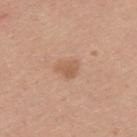<record>
  <biopsy_status>not biopsied; imaged during a skin examination</biopsy_status>
  <lighting>white-light</lighting>
  <patient>
    <sex>male</sex>
    <age_approx>25</age_approx>
  </patient>
  <image>
    <source>total-body photography crop</source>
    <field_of_view_mm>15</field_of_view_mm>
  </image>
  <site>back</site>
</record>